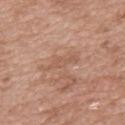{
  "biopsy_status": "not biopsied; imaged during a skin examination",
  "lesion_size": {
    "long_diameter_mm_approx": 3.5
  },
  "patient": {
    "sex": "male",
    "age_approx": 50
  },
  "image": {
    "source": "total-body photography crop",
    "field_of_view_mm": 15
  },
  "automated_metrics": {
    "area_mm2_approx": 3.0,
    "eccentricity": 0.95,
    "shape_asymmetry": 0.4,
    "cielab_L": 56,
    "cielab_a": 20,
    "cielab_b": 30,
    "vs_skin_darker_L": 6.0,
    "lesion_detection_confidence_0_100": 95
  },
  "site": "mid back"
}